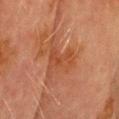The lesion was tiled from a total-body skin photograph and was not biopsied.
From the head or neck.
This is a cross-polarized tile.
Cropped from a whole-body photographic skin survey; the tile spans about 15 mm.
An algorithmic analysis of the crop reported an outline eccentricity of about 0.65 (0 = round, 1 = elongated) and a shape-asymmetry score of about 0.6 (0 = symmetric). The software also gave an automated nevus-likeness rating near 0 out of 100 and lesion-presence confidence of about 100/100.
The lesion's longest dimension is about 5.5 mm.
A male patient aged 68–72.